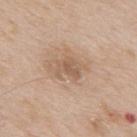Q: Was a biopsy performed?
A: catalogued during a skin exam; not biopsied
Q: What is the anatomic site?
A: the back
Q: How was this image acquired?
A: total-body-photography crop, ~15 mm field of view
Q: Illumination type?
A: white-light
Q: How large is the lesion?
A: ≈3 mm
Q: What are the patient's age and sex?
A: male, roughly 65 years of age
Q: What did automated image analysis measure?
A: an area of roughly 5.5 mm²; an average lesion color of about L≈57 a*≈17 b*≈30 (CIELAB), roughly 8 lightness units darker than nearby skin, and a normalized lesion–skin contrast near 5.5; a border-irregularity index near 5/10, a within-lesion color-variation index near 3/10, and peripheral color asymmetry of about 1